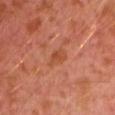Part of a total-body skin-imaging series; this lesion was reviewed on a skin check and was not flagged for biopsy. A close-up tile cropped from a whole-body skin photograph, about 15 mm across. A male subject, aged approximately 30. Located on the left upper arm.